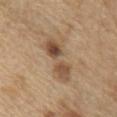{"lesion_size": {"long_diameter_mm_approx": 5.5}, "site": "arm", "automated_metrics": {"cielab_L": 51, "cielab_a": 16, "cielab_b": 31, "vs_skin_darker_L": 11.0, "vs_skin_contrast_norm": 8.0}, "lighting": "white-light", "image": {"source": "total-body photography crop", "field_of_view_mm": 15}, "patient": {"sex": "male", "age_approx": 70}}